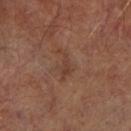{
  "biopsy_status": "not biopsied; imaged during a skin examination",
  "image": {
    "source": "total-body photography crop",
    "field_of_view_mm": 15
  },
  "automated_metrics": {
    "cielab_L": 38,
    "cielab_a": 19,
    "cielab_b": 26,
    "vs_skin_darker_L": 6.0,
    "vs_skin_contrast_norm": 5.0
  },
  "site": "right lower leg",
  "lesion_size": {
    "long_diameter_mm_approx": 4.0
  },
  "lighting": "cross-polarized",
  "patient": {
    "sex": "male",
    "age_approx": 65
  }
}